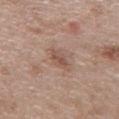The lesion was tiled from a total-body skin photograph and was not biopsied.
The recorded lesion diameter is about 3 mm.
A roughly 15 mm field-of-view crop from a total-body skin photograph.
Automated tile analysis of the lesion measured a footprint of about 3.5 mm², an eccentricity of roughly 0.9, and a symmetry-axis asymmetry near 0.25. The analysis additionally found a mean CIELAB color near L≈52 a*≈19 b*≈26 and a normalized border contrast of about 6.
The lesion is on the chest.
The patient is a female about 40 years old.
Captured under white-light illumination.